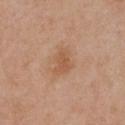Imaged during a routine full-body skin examination; the lesion was not biopsied and no histopathology is available.